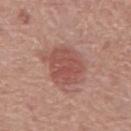Notes:
– follow-up — catalogued during a skin exam; not biopsied
– subject — male, aged 73–77
– imaging modality — 15 mm crop, total-body photography
– lesion diameter — ≈5 mm
– location — the back